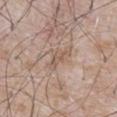The lesion was photographed on a routine skin check and not biopsied; there is no pathology result. This image is a 15 mm lesion crop taken from a total-body photograph. A male subject aged 58–62. The tile uses white-light illumination. On the abdomen. The recorded lesion diameter is about 3.5 mm.